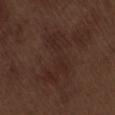biopsy_status: not biopsied; imaged during a skin examination
automated_metrics:
  cielab_L: 25
  cielab_a: 18
  cielab_b: 21
  vs_skin_darker_L: 4.0
  vs_skin_contrast_norm: 5.0
  border_irregularity_0_10: 9.0
  color_variation_0_10: 3.0
  nevus_likeness_0_100: 0
  lesion_detection_confidence_0_100: 100
site: lower back
image:
  source: total-body photography crop
  field_of_view_mm: 15
lesion_size:
  long_diameter_mm_approx: 7.0
patient:
  sex: male
  age_approx: 70
lighting: white-light